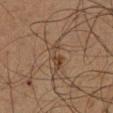Recorded during total-body skin imaging; not selected for excision or biopsy.
Cropped from a whole-body photographic skin survey; the tile spans about 15 mm.
The patient is a male aged 63–67.
The lesion is on the leg.